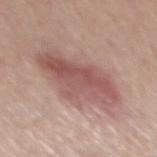Impression:
The lesion was tiled from a total-body skin photograph and was not biopsied.
Acquisition and patient details:
A male patient in their mid-50s. Automated image analysis of the tile measured an automated nevus-likeness rating near 90 out of 100 and lesion-presence confidence of about 100/100. On the mid back. Cropped from a whole-body photographic skin survey; the tile spans about 15 mm.